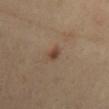workup: imaged on a skin check; not biopsied | location: the mid back | subject: female, aged around 40 | acquisition: 15 mm crop, total-body photography.